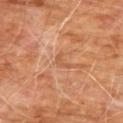Captured during whole-body skin photography for melanoma surveillance; the lesion was not biopsied. A male patient, roughly 60 years of age. The lesion is located on the front of the torso. A 15 mm crop from a total-body photograph taken for skin-cancer surveillance.